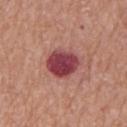{
  "lesion_size": {
    "long_diameter_mm_approx": 4.5
  },
  "site": "left upper arm",
  "image": {
    "source": "total-body photography crop",
    "field_of_view_mm": 15
  },
  "lighting": "white-light",
  "patient": {
    "sex": "male",
    "age_approx": 65
  }
}